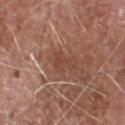A roughly 15 mm field-of-view crop from a total-body skin photograph.
A male patient, aged around 75.
Longest diameter approximately 3 mm.
Captured under white-light illumination.
On the head or neck.